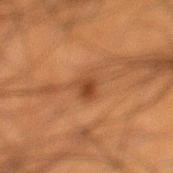The lesion was photographed on a routine skin check and not biopsied; there is no pathology result. Cropped from a total-body skin-imaging series; the visible field is about 15 mm. This is a cross-polarized tile. A male patient, approximately 50 years of age. The lesion is located on the left lower leg. About 3 mm across.